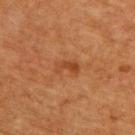The lesion was tiled from a total-body skin photograph and was not biopsied. An algorithmic analysis of the crop reported a lesion color around L≈39 a*≈24 b*≈34 in CIELAB, about 7 CIELAB-L* units darker than the surrounding skin, and a normalized border contrast of about 6. The software also gave a nevus-likeness score of about 10/100 and a detector confidence of about 100 out of 100 that the crop contains a lesion. From the upper back. The tile uses cross-polarized illumination. Longest diameter approximately 3 mm. A lesion tile, about 15 mm wide, cut from a 3D total-body photograph. The subject is a male aged 63–67.